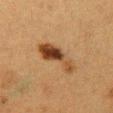workup: catalogued during a skin exam; not biopsied
automated metrics: a footprint of about 10 mm² and an outline eccentricity of about 0.95 (0 = round, 1 = elongated); a lesion color around L≈36 a*≈18 b*≈31 in CIELAB and a lesion–skin lightness drop of about 13; a border-irregularity index near 6/10 and a color-variation rating of about 6/10; an automated nevus-likeness rating near 100 out of 100 and a detector confidence of about 100 out of 100 that the crop contains a lesion
subject: female, aged approximately 40
lesion diameter: ~6 mm (longest diameter)
imaging modality: 15 mm crop, total-body photography
lighting: cross-polarized illumination
body site: the front of the torso